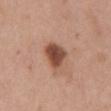follow-up: no biopsy performed (imaged during a skin exam)
site: the front of the torso
image source: 15 mm crop, total-body photography
illumination: white-light
subject: female, aged 58–62
image-analysis metrics: a footprint of about 7.5 mm², an outline eccentricity of about 0.6 (0 = round, 1 = elongated), and a symmetry-axis asymmetry near 0.25; an average lesion color of about L≈47 a*≈23 b*≈29 (CIELAB), roughly 16 lightness units darker than nearby skin, and a normalized border contrast of about 11; a border-irregularity index near 2.5/10, internal color variation of about 3.5 on a 0–10 scale, and peripheral color asymmetry of about 1; a nevus-likeness score of about 100/100 and a lesion-detection confidence of about 100/100